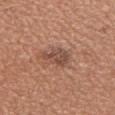Case summary:
* workup: no biopsy performed (imaged during a skin exam)
* patient: female, aged approximately 25
* imaging modality: ~15 mm tile from a whole-body skin photo
* site: the right upper arm